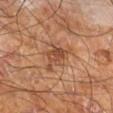No biopsy was performed on this lesion — it was imaged during a full skin examination and was not determined to be concerning.
A roughly 15 mm field-of-view crop from a total-body skin photograph.
Longest diameter approximately 4.5 mm.
On the right leg.
This is a cross-polarized tile.
An algorithmic analysis of the crop reported a lesion area of about 7 mm² and a symmetry-axis asymmetry near 0.45. The software also gave roughly 9 lightness units darker than nearby skin and a normalized lesion–skin contrast near 7. It also reported a border-irregularity rating of about 6/10 and a color-variation rating of about 4/10.
A male patient, aged 58 to 62.